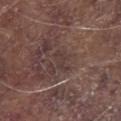{
  "biopsy_status": "not biopsied; imaged during a skin examination",
  "image": {
    "source": "total-body photography crop",
    "field_of_view_mm": 15
  },
  "lighting": "white-light",
  "lesion_size": {
    "long_diameter_mm_approx": 2.5
  },
  "site": "chest",
  "patient": {
    "sex": "male",
    "age_approx": 80
  }
}